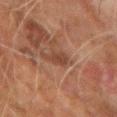{"biopsy_status": "not biopsied; imaged during a skin examination", "site": "left forearm", "image": {"source": "total-body photography crop", "field_of_view_mm": 15}, "patient": {"sex": "male", "age_approx": 60}}